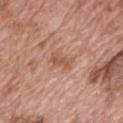notes: catalogued during a skin exam; not biopsied | location: the mid back | patient: male, aged approximately 70 | image source: total-body-photography crop, ~15 mm field of view.